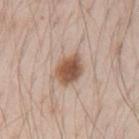The lesion was tiled from a total-body skin photograph and was not biopsied.
From the left upper arm.
Imaged with white-light lighting.
The subject is a male aged around 70.
A lesion tile, about 15 mm wide, cut from a 3D total-body photograph.